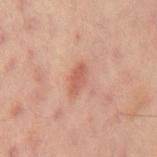Q: Was a biopsy performed?
A: imaged on a skin check; not biopsied
Q: What is the imaging modality?
A: total-body-photography crop, ~15 mm field of view
Q: What did automated image analysis measure?
A: an average lesion color of about L≈53 a*≈25 b*≈29 (CIELAB) and a lesion-to-skin contrast of about 6.5 (normalized; higher = more distinct); a border-irregularity index near 3/10 and a peripheral color-asymmetry measure near 0.5
Q: Lesion size?
A: ≈3 mm
Q: Where on the body is the lesion?
A: the mid back
Q: What are the patient's age and sex?
A: male, aged 58–62